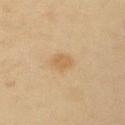Q: Was a biopsy performed?
A: imaged on a skin check; not biopsied
Q: Lesion location?
A: the left upper arm
Q: How was the tile lit?
A: cross-polarized
Q: Who is the patient?
A: female, aged around 55
Q: Automated lesion metrics?
A: an area of roughly 5 mm²; an average lesion color of about L≈54 a*≈14 b*≈34 (CIELAB) and roughly 6 lightness units darker than nearby skin
Q: What is the lesion's diameter?
A: ≈3 mm
Q: What kind of image is this?
A: ~15 mm crop, total-body skin-cancer survey